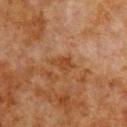Captured during whole-body skin photography for melanoma surveillance; the lesion was not biopsied. A roughly 15 mm field-of-view crop from a total-body skin photograph. A male subject approximately 80 years of age. An algorithmic analysis of the crop reported a lesion area of about 3.5 mm², an eccentricity of roughly 0.75, and two-axis asymmetry of about 0.3. It also reported a within-lesion color-variation index near 1/10. It also reported a classifier nevus-likeness of about 0/100 and a detector confidence of about 100 out of 100 that the crop contains a lesion. Approximately 3 mm at its widest. From the right upper arm.